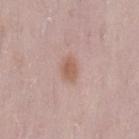Findings:
* biopsy status: no biopsy performed (imaged during a skin exam)
* site: the left thigh
* lighting: white-light
* patient: female, in their 30s
* imaging modality: ~15 mm tile from a whole-body skin photo
* lesion size: ~2.5 mm (longest diameter)